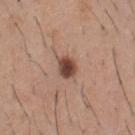The subject is a male approximately 60 years of age.
Imaged with white-light lighting.
An algorithmic analysis of the crop reported roughly 15 lightness units darker than nearby skin and a normalized border contrast of about 11. The analysis additionally found a classifier nevus-likeness of about 95/100 and a detector confidence of about 100 out of 100 that the crop contains a lesion.
On the front of the torso.
A region of skin cropped from a whole-body photographic capture, roughly 15 mm wide.